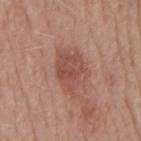{"biopsy_status": "not biopsied; imaged during a skin examination", "patient": {"sex": "male", "age_approx": 50}, "image": {"source": "total-body photography crop", "field_of_view_mm": 15}, "site": "back", "lesion_size": {"long_diameter_mm_approx": 5.5}, "automated_metrics": {"eccentricity": 0.75, "shape_asymmetry": 0.3, "cielab_L": 50, "cielab_a": 24, "cielab_b": 26, "vs_skin_darker_L": 9.0, "vs_skin_contrast_norm": 6.5, "nevus_likeness_0_100": 60, "lesion_detection_confidence_0_100": 100}, "lighting": "white-light"}